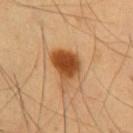workup: catalogued during a skin exam; not biopsied